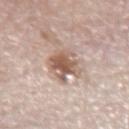Assessment: Recorded during total-body skin imaging; not selected for excision or biopsy. Context: Longest diameter approximately 5 mm. This image is a 15 mm lesion crop taken from a total-body photograph. The tile uses white-light illumination. An algorithmic analysis of the crop reported a lesion area of about 11 mm², an outline eccentricity of about 0.75 (0 = round, 1 = elongated), and a shape-asymmetry score of about 0.3 (0 = symmetric). The analysis additionally found an average lesion color of about L≈59 a*≈17 b*≈26 (CIELAB), a lesion–skin lightness drop of about 13, and a normalized border contrast of about 8.5. The software also gave border irregularity of about 4 on a 0–10 scale, internal color variation of about 6.5 on a 0–10 scale, and peripheral color asymmetry of about 2. The lesion is on the right forearm. The patient is a female in their mid- to late 60s.